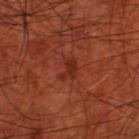<record>
  <lighting>cross-polarized</lighting>
  <automated_metrics>
    <eccentricity>0.7</eccentricity>
    <cielab_L>29</cielab_L>
    <cielab_a>30</cielab_a>
    <cielab_b>30</cielab_b>
    <border_irregularity_0_10>5.0</border_irregularity_0_10>
    <color_variation_0_10>1.5</color_variation_0_10>
    <peripheral_color_asymmetry>0.5</peripheral_color_asymmetry>
    <nevus_likeness_0_100>0</nevus_likeness_0_100>
    <lesion_detection_confidence_0_100>100</lesion_detection_confidence_0_100>
  </automated_metrics>
  <lesion_size>
    <long_diameter_mm_approx>2.5</long_diameter_mm_approx>
  </lesion_size>
  <site>left thigh</site>
  <image>
    <source>total-body photography crop</source>
    <field_of_view_mm>15</field_of_view_mm>
  </image>
  <patient>
    <sex>male</sex>
    <age_approx>70</age_approx>
  </patient>
</record>